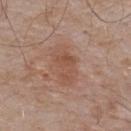Captured during whole-body skin photography for melanoma surveillance; the lesion was not biopsied.
The lesion is located on the upper back.
Longest diameter approximately 4.5 mm.
A 15 mm crop from a total-body photograph taken for skin-cancer surveillance.
Imaged with white-light lighting.
A male subject, in their mid-50s.